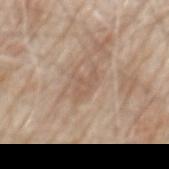<lesion>
  <biopsy_status>not biopsied; imaged during a skin examination</biopsy_status>
  <lighting>white-light</lighting>
  <patient>
    <sex>male</sex>
    <age_approx>80</age_approx>
  </patient>
  <image>
    <source>total-body photography crop</source>
    <field_of_view_mm>15</field_of_view_mm>
  </image>
  <site>mid back</site>
  <lesion_size>
    <long_diameter_mm_approx>3.5</long_diameter_mm_approx>
  </lesion_size>
</lesion>